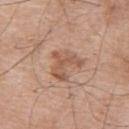* follow-up — imaged on a skin check; not biopsied
* body site — the upper back
* illumination — white-light illumination
* imaging modality — 15 mm crop, total-body photography
* automated lesion analysis — a footprint of about 8.5 mm², an eccentricity of roughly 0.5, and a symmetry-axis asymmetry near 0.65; a lesion color around L≈55 a*≈20 b*≈30 in CIELAB, about 9 CIELAB-L* units darker than the surrounding skin, and a lesion-to-skin contrast of about 6.5 (normalized; higher = more distinct); a border-irregularity index near 8/10; a classifier nevus-likeness of about 5/100 and lesion-presence confidence of about 100/100
* patient — male, in their mid-60s
* lesion diameter — ~4 mm (longest diameter)